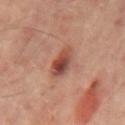| field | value |
|---|---|
| follow-up | total-body-photography surveillance lesion; no biopsy |
| anatomic site | the back |
| acquisition | total-body-photography crop, ~15 mm field of view |
| lesion size | about 3.5 mm |
| subject | male, in their mid-60s |
| lighting | cross-polarized |